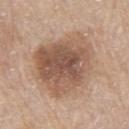Clinical impression: The lesion was tiled from a total-body skin photograph and was not biopsied. Context: A male subject roughly 80 years of age. Located on the left upper arm. The tile uses white-light illumination. A roughly 15 mm field-of-view crop from a total-body skin photograph. The recorded lesion diameter is about 7 mm.